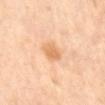follow-up = imaged on a skin check; not biopsied | lighting = cross-polarized illumination | imaging modality = ~15 mm crop, total-body skin-cancer survey | anatomic site = the mid back | lesion diameter = ≈2.5 mm | automated lesion analysis = an eccentricity of roughly 0.6 and a symmetry-axis asymmetry near 0.2; about 9 CIELAB-L* units darker than the surrounding skin and a normalized border contrast of about 6.5; a color-variation rating of about 3/10 and a peripheral color-asymmetry measure near 1 | subject = male, aged 58 to 62.